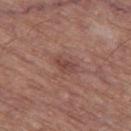Notes:
* biopsy status: total-body-photography surveillance lesion; no biopsy